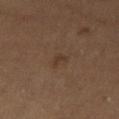Case summary:
- workup: no biopsy performed (imaged during a skin exam)
- imaging modality: 15 mm crop, total-body photography
- site: the leg
- automated lesion analysis: a mean CIELAB color near L≈32 a*≈16 b*≈25 and a normalized lesion–skin contrast near 6; a border-irregularity rating of about 4/10, a color-variation rating of about 0/10, and peripheral color asymmetry of about 0
- diameter: about 1.5 mm
- illumination: cross-polarized illumination
- subject: female, in their mid-60s